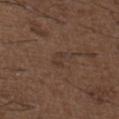biopsy status — imaged on a skin check; not biopsied
image source — 15 mm crop, total-body photography
lighting — white-light illumination
subject — male, aged around 50
anatomic site — the upper back
lesion size — about 2.5 mm
TBP lesion metrics — an area of roughly 2.5 mm², a shape eccentricity near 0.9, and a shape-asymmetry score of about 0.45 (0 = symmetric); a mean CIELAB color near L≈34 a*≈15 b*≈23, a lesion–skin lightness drop of about 5, and a normalized lesion–skin contrast near 5; lesion-presence confidence of about 100/100